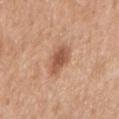Part of a total-body skin-imaging series; this lesion was reviewed on a skin check and was not flagged for biopsy. The lesion is located on the right upper arm. A female patient, aged around 50. A 15 mm close-up tile from a total-body photography series done for melanoma screening.